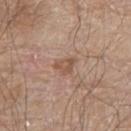No biopsy was performed on this lesion — it was imaged during a full skin examination and was not determined to be concerning.
The lesion is located on the arm.
The total-body-photography lesion software estimated an eccentricity of roughly 0.7. It also reported internal color variation of about 2.5 on a 0–10 scale and radial color variation of about 1.
Longest diameter approximately 3 mm.
A region of skin cropped from a whole-body photographic capture, roughly 15 mm wide.
A male subject, aged 78 to 82.